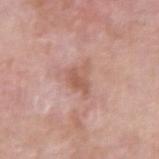notes: imaged on a skin check; not biopsied
subject: male, in their mid- to late 60s
lesion size: ~3.5 mm (longest diameter)
lighting: white-light
imaging modality: ~15 mm crop, total-body skin-cancer survey
body site: the right upper arm
TBP lesion metrics: a within-lesion color-variation index near 3.5/10 and a peripheral color-asymmetry measure near 1